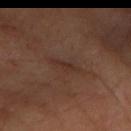biopsy status: catalogued during a skin exam; not biopsied
patient: female
acquisition: total-body-photography crop, ~15 mm field of view
location: the left forearm